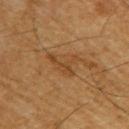follow-up: total-body-photography surveillance lesion; no biopsy
anatomic site: the left upper arm
image: ~15 mm crop, total-body skin-cancer survey
subject: male, aged 58–62
illumination: cross-polarized illumination